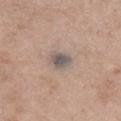– biopsy status: no biopsy performed (imaged during a skin exam)
– size: ~3 mm (longest diameter)
– subject: female, roughly 65 years of age
– automated metrics: an area of roughly 5 mm² and a shape-asymmetry score of about 0.2 (0 = symmetric); a mean CIELAB color near L≈54 a*≈9 b*≈18, a lesion–skin lightness drop of about 11, and a lesion-to-skin contrast of about 9 (normalized; higher = more distinct); a border-irregularity index near 2/10, a color-variation rating of about 4/10, and peripheral color asymmetry of about 1
– body site: the chest
– imaging modality: ~15 mm crop, total-body skin-cancer survey
– tile lighting: white-light